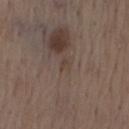Captured during whole-body skin photography for melanoma surveillance; the lesion was not biopsied. A male subject, aged 68–72. A roughly 15 mm field-of-view crop from a total-body skin photograph. Measured at roughly 1.5 mm in maximum diameter. The tile uses white-light illumination. The lesion is located on the back. The total-body-photography lesion software estimated a footprint of about 1.5 mm², an eccentricity of roughly 0.75, and a shape-asymmetry score of about 0.35 (0 = symmetric). It also reported about 5 CIELAB-L* units darker than the surrounding skin and a normalized lesion–skin contrast near 5. The software also gave a border-irregularity index near 3/10, internal color variation of about 0 on a 0–10 scale, and a peripheral color-asymmetry measure near 0. And it measured a lesion-detection confidence of about 55/100.